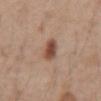Clinical summary:
Longest diameter approximately 3 mm. The tile uses white-light illumination. This image is a 15 mm lesion crop taken from a total-body photograph. A male subject, about 60 years old. The lesion is located on the chest. Automated image analysis of the tile measured a footprint of about 5.5 mm², an outline eccentricity of about 0.8 (0 = round, 1 = elongated), and a symmetry-axis asymmetry near 0.25. The software also gave a mean CIELAB color near L≈49 a*≈21 b*≈28, roughly 13 lightness units darker than nearby skin, and a normalized lesion–skin contrast near 9.5.